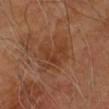About 4.5 mm across.
A male subject, approximately 70 years of age.
A 15 mm close-up extracted from a 3D total-body photography capture.
This is a cross-polarized tile.
On the arm.
An algorithmic analysis of the crop reported border irregularity of about 5.5 on a 0–10 scale, a color-variation rating of about 3.5/10, and peripheral color asymmetry of about 1.